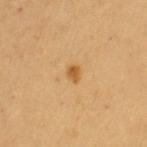| field | value |
|---|---|
| biopsy status | imaged on a skin check; not biopsied |
| imaging modality | 15 mm crop, total-body photography |
| subject | female, approximately 65 years of age |
| anatomic site | the right upper arm |
| lesion size | ≈2 mm |
| TBP lesion metrics | a classifier nevus-likeness of about 90/100 |
| lighting | cross-polarized |